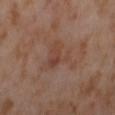Q: Was a biopsy performed?
A: total-body-photography surveillance lesion; no biopsy
Q: What kind of image is this?
A: 15 mm crop, total-body photography
Q: Lesion size?
A: about 5.5 mm
Q: How was the tile lit?
A: cross-polarized illumination
Q: Where on the body is the lesion?
A: the right thigh
Q: Who is the patient?
A: female, roughly 55 years of age